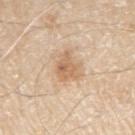• notes — no biopsy performed (imaged during a skin exam)
• image source — total-body-photography crop, ~15 mm field of view
• size — ~3.5 mm (longest diameter)
• site — the left upper arm
• patient — male, roughly 80 years of age